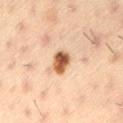patient: male, approximately 55 years of age | size: ~3 mm (longest diameter) | acquisition: 15 mm crop, total-body photography | location: the lower back | lighting: cross-polarized.